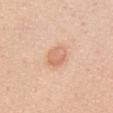illumination=white-light; subject=female, aged approximately 40; anatomic site=the chest; lesion size=about 3 mm; automated lesion analysis=a footprint of about 6 mm², an eccentricity of roughly 0.7, and two-axis asymmetry of about 0.3; acquisition=15 mm crop, total-body photography.